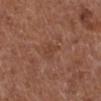follow-up: catalogued during a skin exam; not biopsied
anatomic site: the right lower leg
TBP lesion metrics: an average lesion color of about L≈42 a*≈22 b*≈29 (CIELAB) and a lesion–skin lightness drop of about 5; a nevus-likeness score of about 0/100 and lesion-presence confidence of about 100/100
lesion diameter: about 2.5 mm
lighting: white-light
image source: 15 mm crop, total-body photography
subject: male, aged 73–77